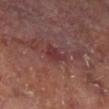notes: catalogued during a skin exam; not biopsied | tile lighting: cross-polarized | subject: male, aged around 65 | image: 15 mm crop, total-body photography | anatomic site: the right lower leg | size: ~2.5 mm (longest diameter) | automated metrics: a footprint of about 4 mm², an eccentricity of roughly 0.45, and a symmetry-axis asymmetry near 0.35; a lesion color around L≈33 a*≈25 b*≈19 in CIELAB, roughly 7 lightness units darker than nearby skin, and a lesion-to-skin contrast of about 6.5 (normalized; higher = more distinct); a peripheral color-asymmetry measure near 0.5; a classifier nevus-likeness of about 0/100 and a lesion-detection confidence of about 100/100.